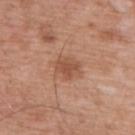The lesion was tiled from a total-body skin photograph and was not biopsied.
The subject is a male aged 58–62.
A 15 mm crop from a total-body photograph taken for skin-cancer surveillance.
The lesion is located on the chest.